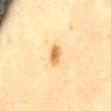Assessment: The lesion was photographed on a routine skin check and not biopsied; there is no pathology result. Context: A region of skin cropped from a whole-body photographic capture, roughly 15 mm wide. A female subject, aged 53 to 57. From the chest.